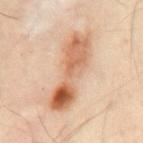{"biopsy_status": "not biopsied; imaged during a skin examination", "image": {"source": "total-body photography crop", "field_of_view_mm": 15}, "automated_metrics": {"area_mm2_approx": 21.0, "eccentricity": 0.95, "shape_asymmetry": 0.3, "border_irregularity_0_10": 4.5, "color_variation_0_10": 10.0, "peripheral_color_asymmetry": 3.5, "nevus_likeness_0_100": 60, "lesion_detection_confidence_0_100": 100}, "lighting": "cross-polarized", "patient": {"sex": "male", "age_approx": 50}, "site": "mid back"}